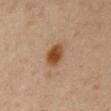biopsy status: no biopsy performed (imaged during a skin exam)
anatomic site: the abdomen
patient: male, roughly 60 years of age
acquisition: ~15 mm tile from a whole-body skin photo